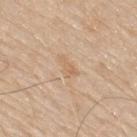{
  "patient": {
    "sex": "male",
    "age_approx": 80
  },
  "image": {
    "source": "total-body photography crop",
    "field_of_view_mm": 15
  },
  "lighting": "white-light",
  "lesion_size": {
    "long_diameter_mm_approx": 3.0
  },
  "site": "mid back",
  "automated_metrics": {
    "eccentricity": 0.85,
    "shape_asymmetry": 0.45,
    "border_irregularity_0_10": 5.0,
    "color_variation_0_10": 1.0,
    "lesion_detection_confidence_0_100": 100
  }
}